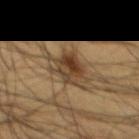follow-up=imaged on a skin check; not biopsied
lighting=cross-polarized
anatomic site=the abdomen
acquisition=total-body-photography crop, ~15 mm field of view
size=≈5 mm
patient=male, roughly 60 years of age